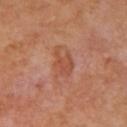{
  "biopsy_status": "not biopsied; imaged during a skin examination",
  "lighting": "cross-polarized",
  "image": {
    "source": "total-body photography crop",
    "field_of_view_mm": 15
  },
  "site": "right upper arm",
  "patient": {
    "sex": "female",
    "age_approx": 55
  }
}